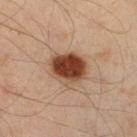Approximately 4.5 mm at its widest. A 15 mm crop from a total-body photograph taken for skin-cancer surveillance. Imaged with cross-polarized lighting. The subject is a male in their mid-40s. Automated image analysis of the tile measured a lesion area of about 13 mm² and a shape eccentricity near 0.6. The software also gave a mean CIELAB color near L≈37 a*≈20 b*≈27, about 16 CIELAB-L* units darker than the surrounding skin, and a normalized border contrast of about 13. It also reported border irregularity of about 1.5 on a 0–10 scale, internal color variation of about 6 on a 0–10 scale, and peripheral color asymmetry of about 2. And it measured a classifier nevus-likeness of about 100/100 and lesion-presence confidence of about 100/100. The lesion is on the right lower leg.